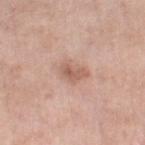Q: Was this lesion biopsied?
A: total-body-photography surveillance lesion; no biopsy
Q: How large is the lesion?
A: ≈3 mm
Q: How was the tile lit?
A: white-light
Q: What is the anatomic site?
A: the right thigh
Q: Patient demographics?
A: female, about 55 years old
Q: What did automated image analysis measure?
A: a footprint of about 4.5 mm², an outline eccentricity of about 0.75 (0 = round, 1 = elongated), and a shape-asymmetry score of about 0.35 (0 = symmetric); a border-irregularity rating of about 3.5/10 and radial color variation of about 1.5; an automated nevus-likeness rating near 0 out of 100 and a lesion-detection confidence of about 100/100
Q: What kind of image is this?
A: total-body-photography crop, ~15 mm field of view